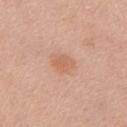{"biopsy_status": "not biopsied; imaged during a skin examination", "lighting": "white-light", "site": "front of the torso", "automated_metrics": {"cielab_L": 62, "cielab_a": 23, "cielab_b": 33, "vs_skin_contrast_norm": 5.5, "nevus_likeness_0_100": 40, "lesion_detection_confidence_0_100": 100}, "lesion_size": {"long_diameter_mm_approx": 2.5}, "image": {"source": "total-body photography crop", "field_of_view_mm": 15}, "patient": {"sex": "male", "age_approx": 70}}